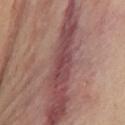This lesion was catalogued during total-body skin photography and was not selected for biopsy. Approximately 3 mm at its widest. Captured under cross-polarized illumination. A roughly 15 mm field-of-view crop from a total-body skin photograph. A female subject, in their 60s. The lesion-visualizer software estimated internal color variation of about 0 on a 0–10 scale. The lesion is located on the mid back.